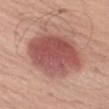Case summary:
- biopsy status: imaged on a skin check; not biopsied
- acquisition: ~15 mm tile from a whole-body skin photo
- patient: male, aged 68–72
- site: the leg